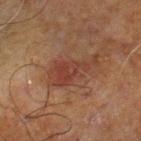{
  "biopsy_status": "not biopsied; imaged during a skin examination",
  "site": "right lower leg",
  "patient": {
    "sex": "male",
    "age_approx": 70
  },
  "lighting": "cross-polarized",
  "automated_metrics": {
    "area_mm2_approx": 11.0,
    "eccentricity": 0.9,
    "shape_asymmetry": 0.45,
    "border_irregularity_0_10": 7.0,
    "peripheral_color_asymmetry": 1.0
  },
  "image": {
    "source": "total-body photography crop",
    "field_of_view_mm": 15
  }
}